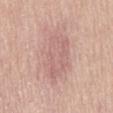Impression: Captured during whole-body skin photography for melanoma surveillance; the lesion was not biopsied. Acquisition and patient details: A male patient, aged 53 to 57. Imaged with white-light lighting. A 15 mm close-up extracted from a 3D total-body photography capture.